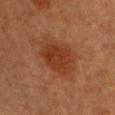  biopsy_status: not biopsied; imaged during a skin examination
  image:
    source: total-body photography crop
    field_of_view_mm: 15
  lighting: cross-polarized
  patient:
    sex: female
    age_approx: 40
  site: chest
  lesion_size:
    long_diameter_mm_approx: 6.0
  automated_metrics:
    vs_skin_contrast_norm: 7.5
    nevus_likeness_0_100: 95
    lesion_detection_confidence_0_100: 100